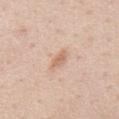Q: Was a biopsy performed?
A: imaged on a skin check; not biopsied
Q: Lesion size?
A: ~2.5 mm (longest diameter)
Q: What is the imaging modality?
A: ~15 mm crop, total-body skin-cancer survey
Q: Illumination type?
A: white-light illumination
Q: Lesion location?
A: the back
Q: Patient demographics?
A: male, roughly 60 years of age